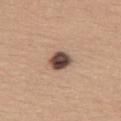Impression: The lesion was tiled from a total-body skin photograph and was not biopsied. Context: A 15 mm crop from a total-body photograph taken for skin-cancer surveillance. On the right thigh. A female patient aged around 60.